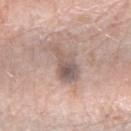workup = total-body-photography surveillance lesion; no biopsy | subject = female, in their 40s | image-analysis metrics = border irregularity of about 4.5 on a 0–10 scale and a peripheral color-asymmetry measure near 2; a classifier nevus-likeness of about 5/100 and a detector confidence of about 90 out of 100 that the crop contains a lesion | image = ~15 mm tile from a whole-body skin photo | anatomic site = the right forearm | diameter = ≈4.5 mm.